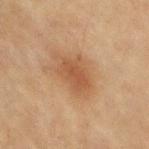Clinical impression: Recorded during total-body skin imaging; not selected for excision or biopsy. Context: This is a cross-polarized tile. A female patient roughly 80 years of age. Longest diameter approximately 4 mm. A close-up tile cropped from a whole-body skin photograph, about 15 mm across. On the right upper arm.